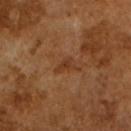notes=imaged on a skin check; not biopsied | image source=~15 mm tile from a whole-body skin photo | subject=male, in their mid- to late 60s.